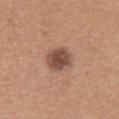* workup · catalogued during a skin exam; not biopsied
* size · ≈3.5 mm
* anatomic site · the upper back
* image source · total-body-photography crop, ~15 mm field of view
* patient · female, aged around 30